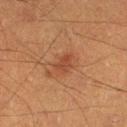workup: catalogued during a skin exam; not biopsied
anatomic site: the left lower leg
subject: male, aged around 40
image source: ~15 mm tile from a whole-body skin photo
lesion diameter: about 2.5 mm